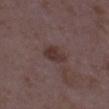{
  "biopsy_status": "not biopsied; imaged during a skin examination",
  "image": {
    "source": "total-body photography crop",
    "field_of_view_mm": 15
  },
  "patient": {
    "sex": "female",
    "age_approx": 35
  },
  "site": "left thigh",
  "lighting": "white-light"
}